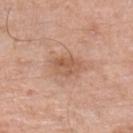Q: Is there a histopathology result?
A: total-body-photography surveillance lesion; no biopsy
Q: What lighting was used for the tile?
A: white-light illumination
Q: What kind of image is this?
A: ~15 mm tile from a whole-body skin photo
Q: What are the patient's age and sex?
A: male, in their 80s
Q: Lesion location?
A: the left lower leg
Q: What is the lesion's diameter?
A: ≈4 mm